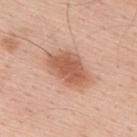Q: Is there a histopathology result?
A: no biopsy performed (imaged during a skin exam)
Q: Patient demographics?
A: male, aged 53 to 57
Q: How was this image acquired?
A: total-body-photography crop, ~15 mm field of view
Q: Where on the body is the lesion?
A: the back
Q: Automated lesion metrics?
A: a lesion area of about 15 mm² and two-axis asymmetry of about 0.2; an automated nevus-likeness rating near 95 out of 100